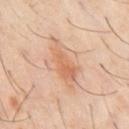<case>
<biopsy_status>not biopsied; imaged during a skin examination</biopsy_status>
<patient>
  <sex>male</sex>
  <age_approx>60</age_approx>
</patient>
<image>
  <source>total-body photography crop</source>
  <field_of_view_mm>15</field_of_view_mm>
</image>
<lighting>cross-polarized</lighting>
<lesion_size>
  <long_diameter_mm_approx>5.0</long_diameter_mm_approx>
</lesion_size>
<site>abdomen</site>
<automated_metrics>
  <area_mm2_approx>8.5</area_mm2_approx>
  <eccentricity>0.9</eccentricity>
  <cielab_L>59</cielab_L>
  <cielab_a>21</cielab_a>
  <cielab_b>32</cielab_b>
  <vs_skin_contrast_norm>6.5</vs_skin_contrast_norm>
  <border_irregularity_0_10>5.0</border_irregularity_0_10>
  <color_variation_0_10>3.0</color_variation_0_10>
  <peripheral_color_asymmetry>1.0</peripheral_color_asymmetry>
</automated_metrics>
</case>